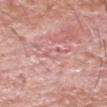<case>
  <site>head or neck</site>
  <lesion_size>
    <long_diameter_mm_approx>2.5</long_diameter_mm_approx>
  </lesion_size>
  <patient>
    <sex>male</sex>
    <age_approx>60</age_approx>
  </patient>
  <lighting>white-light</lighting>
  <image>
    <source>total-body photography crop</source>
    <field_of_view_mm>15</field_of_view_mm>
  </image>
  <diagnosis>
    <histopathology>nodular basal cell carcinoma</histopathology>
    <malignancy>malignant</malignancy>
    <taxonomic_path>Malignant; Malignant adnexal epithelial proliferations - Follicular; Basal cell carcinoma; Basal cell carcinoma, Nodular</taxonomic_path>
  </diagnosis>
</case>